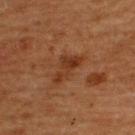Impression: Captured during whole-body skin photography for melanoma surveillance; the lesion was not biopsied. Clinical summary: This is a cross-polarized tile. A roughly 15 mm field-of-view crop from a total-body skin photograph. Located on the back. The subject is a male aged 63–67. Automated tile analysis of the lesion measured a footprint of about 6.5 mm², an outline eccentricity of about 0.9 (0 = round, 1 = elongated), and a shape-asymmetry score of about 0.55 (0 = symmetric). And it measured a lesion color around L≈31 a*≈21 b*≈30 in CIELAB, roughly 7 lightness units darker than nearby skin, and a normalized lesion–skin contrast near 7. The software also gave an automated nevus-likeness rating near 0 out of 100.